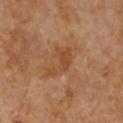{"biopsy_status": "not biopsied; imaged during a skin examination", "patient": {"sex": "female", "age_approx": 50}, "image": {"source": "total-body photography crop", "field_of_view_mm": 15}, "lesion_size": {"long_diameter_mm_approx": 4.5}, "site": "chest"}